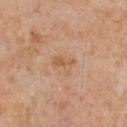Q: Was this lesion biopsied?
A: total-body-photography surveillance lesion; no biopsy
Q: What is the imaging modality?
A: ~15 mm tile from a whole-body skin photo
Q: Illumination type?
A: white-light illumination
Q: Where on the body is the lesion?
A: the chest
Q: Who is the patient?
A: male, in their 60s